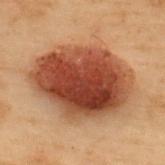notes: total-body-photography surveillance lesion; no biopsy
acquisition: 15 mm crop, total-body photography
body site: the back
subject: male, aged 53 to 57
lesion diameter: ~10 mm (longest diameter)
lighting: cross-polarized illumination